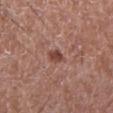- workup: total-body-photography surveillance lesion; no biopsy
- patient: male, roughly 50 years of age
- TBP lesion metrics: a footprint of about 3.5 mm² and a shape-asymmetry score of about 0.25 (0 = symmetric); a lesion color around L≈45 a*≈23 b*≈26 in CIELAB and a lesion–skin lightness drop of about 10; border irregularity of about 2.5 on a 0–10 scale and a within-lesion color-variation index near 2/10
- image source: ~15 mm crop, total-body skin-cancer survey
- body site: the right lower leg